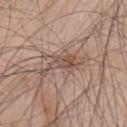Case summary:
• follow-up: no biopsy performed (imaged during a skin exam)
• body site: the front of the torso
• subject: male, roughly 45 years of age
• illumination: white-light
• image source: ~15 mm tile from a whole-body skin photo